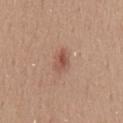Case summary:
- workup — imaged on a skin check; not biopsied
- subject — male, aged around 55
- image-analysis metrics — an area of roughly 5.5 mm² and an eccentricity of roughly 0.8; a lesion color around L≈53 a*≈22 b*≈28 in CIELAB, about 9 CIELAB-L* units darker than the surrounding skin, and a normalized lesion–skin contrast near 6.5; a border-irregularity rating of about 2.5/10, a within-lesion color-variation index near 4.5/10, and peripheral color asymmetry of about 1.5
- size — ~3.5 mm (longest diameter)
- body site — the front of the torso
- acquisition — ~15 mm crop, total-body skin-cancer survey